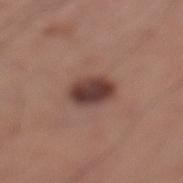biopsy_status: not biopsied; imaged during a skin examination
image:
  source: total-body photography crop
  field_of_view_mm: 15
patient:
  sex: male
  age_approx: 55
site: left lower leg
lesion_size:
  long_diameter_mm_approx: 4.0